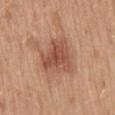Recorded during total-body skin imaging; not selected for excision or biopsy. The recorded lesion diameter is about 4.5 mm. Automated image analysis of the tile measured an area of roughly 14 mm², a shape eccentricity near 0.55, and a symmetry-axis asymmetry near 0.4. The analysis additionally found a mean CIELAB color near L≈52 a*≈24 b*≈30, about 10 CIELAB-L* units darker than the surrounding skin, and a lesion-to-skin contrast of about 7 (normalized; higher = more distinct). The analysis additionally found a classifier nevus-likeness of about 5/100 and lesion-presence confidence of about 100/100. Cropped from a total-body skin-imaging series; the visible field is about 15 mm. The subject is a female roughly 40 years of age. From the mid back.